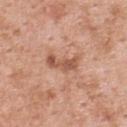Clinical impression:
Imaged during a routine full-body skin examination; the lesion was not biopsied and no histopathology is available.
Clinical summary:
About 4.5 mm across. A female patient aged around 50. A 15 mm close-up tile from a total-body photography series done for melanoma screening. The lesion is located on the upper back. Automated image analysis of the tile measured roughly 11 lightness units darker than nearby skin and a normalized lesion–skin contrast near 7.5.